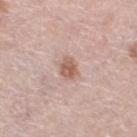This lesion was catalogued during total-body skin photography and was not selected for biopsy.